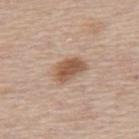biopsy status: no biopsy performed (imaged during a skin exam)
acquisition: ~15 mm crop, total-body skin-cancer survey
subject: male, aged 53–57
body site: the upper back